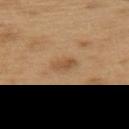follow-up: imaged on a skin check; not biopsied | body site: the upper back | size: about 3 mm | patient: male, aged 68 to 72 | imaging modality: ~15 mm crop, total-body skin-cancer survey | tile lighting: white-light illumination.